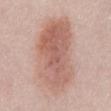Impression: No biopsy was performed on this lesion — it was imaged during a full skin examination and was not determined to be concerning. Context: The lesion is located on the abdomen. Captured under white-light illumination. Cropped from a whole-body photographic skin survey; the tile spans about 15 mm. The subject is a female aged approximately 50. Longest diameter approximately 10 mm.